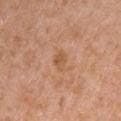Assessment:
This lesion was catalogued during total-body skin photography and was not selected for biopsy.
Background:
On the chest. The subject is a male aged around 65. A region of skin cropped from a whole-body photographic capture, roughly 15 mm wide.